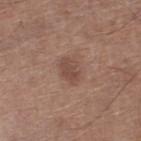Q: Is there a histopathology result?
A: imaged on a skin check; not biopsied
Q: What is the lesion's diameter?
A: ≈3 mm
Q: Patient demographics?
A: male, aged approximately 70
Q: What did automated image analysis measure?
A: an automated nevus-likeness rating near 5 out of 100
Q: Lesion location?
A: the right lower leg
Q: How was this image acquired?
A: ~15 mm tile from a whole-body skin photo